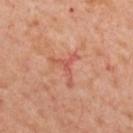Case summary:
• notes — total-body-photography surveillance lesion; no biopsy
• lesion diameter — ≈4 mm
• image — 15 mm crop, total-body photography
• anatomic site — the upper back
• patient — male, aged 53–57
• lighting — cross-polarized